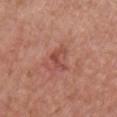<lesion>
  <biopsy_status>not biopsied; imaged during a skin examination</biopsy_status>
  <lighting>white-light</lighting>
  <site>chest</site>
  <patient>
    <sex>female</sex>
    <age_approx>45</age_approx>
  </patient>
  <image>
    <source>total-body photography crop</source>
    <field_of_view_mm>15</field_of_view_mm>
  </image>
  <lesion_size>
    <long_diameter_mm_approx>2.5</long_diameter_mm_approx>
  </lesion_size>
</lesion>